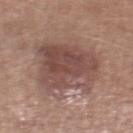  biopsy_status: not biopsied; imaged during a skin examination
  lesion_size:
    long_diameter_mm_approx: 7.5
  site: right lower leg
  image:
    source: total-body photography crop
    field_of_view_mm: 15
  lighting: white-light
  automated_metrics:
    eccentricity: 0.6
    shape_asymmetry: 0.2
    border_irregularity_0_10: 2.5
    color_variation_0_10: 5.0
    peripheral_color_asymmetry: 2.0
    nevus_likeness_0_100: 50
    lesion_detection_confidence_0_100: 100
  patient:
    sex: male
    age_approx: 65